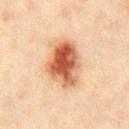The lesion was tiled from a total-body skin photograph and was not biopsied. Located on the chest. Longest diameter approximately 6 mm. A 15 mm close-up tile from a total-body photography series done for melanoma screening. A male patient, aged 38–42. An algorithmic analysis of the crop reported a footprint of about 19 mm², an eccentricity of roughly 0.7, and a symmetry-axis asymmetry near 0.25. And it measured border irregularity of about 2.5 on a 0–10 scale, a color-variation rating of about 10/10, and radial color variation of about 3.5. This is a cross-polarized tile.